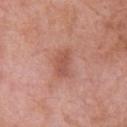{"biopsy_status": "not biopsied; imaged during a skin examination", "lesion_size": {"long_diameter_mm_approx": 3.5}, "automated_metrics": {"border_irregularity_0_10": 2.0, "color_variation_0_10": 2.0, "peripheral_color_asymmetry": 0.5, "nevus_likeness_0_100": 0, "lesion_detection_confidence_0_100": 100}, "patient": {"sex": "male", "age_approx": 70}, "image": {"source": "total-body photography crop", "field_of_view_mm": 15}, "site": "chest"}